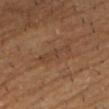The lesion was tiled from a total-body skin photograph and was not biopsied. A close-up tile cropped from a whole-body skin photograph, about 15 mm across. A male subject approximately 85 years of age or older. About 4.5 mm across. The lesion is on the chest.